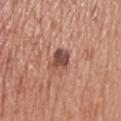Recorded during total-body skin imaging; not selected for excision or biopsy.
A close-up tile cropped from a whole-body skin photograph, about 15 mm across.
From the head or neck.
Imaged with white-light lighting.
A male patient aged 68 to 72.
Longest diameter approximately 3 mm.